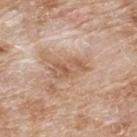<case>
  <biopsy_status>not biopsied; imaged during a skin examination</biopsy_status>
  <lighting>white-light</lighting>
  <site>upper back</site>
  <lesion_size>
    <long_diameter_mm_approx>4.0</long_diameter_mm_approx>
  </lesion_size>
  <image>
    <source>total-body photography crop</source>
    <field_of_view_mm>15</field_of_view_mm>
  </image>
  <patient>
    <sex>male</sex>
    <age_approx>80</age_approx>
  </patient>
</case>